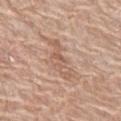The lesion was photographed on a routine skin check and not biopsied; there is no pathology result. A 15 mm crop from a total-body photograph taken for skin-cancer surveillance. A female subject aged 58 to 62. From the left thigh. This is a white-light tile. The recorded lesion diameter is about 5.5 mm.